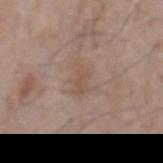Assessment: Recorded during total-body skin imaging; not selected for excision or biopsy. Context: This image is a 15 mm lesion crop taken from a total-body photograph. About 4 mm across. A male subject, aged 63–67. The tile uses white-light illumination. On the front of the torso.